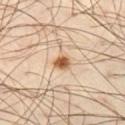Captured during whole-body skin photography for melanoma surveillance; the lesion was not biopsied. A male subject, roughly 50 years of age. Captured under cross-polarized illumination. About 2.5 mm across. A roughly 15 mm field-of-view crop from a total-body skin photograph. The lesion is on the left thigh.